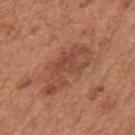* notes — catalogued during a skin exam; not biopsied
* patient — male, in their mid- to late 60s
* body site — the mid back
* image — ~15 mm tile from a whole-body skin photo
* lesion diameter — ~7 mm (longest diameter)
* automated lesion analysis — a lesion area of about 16 mm² and two-axis asymmetry of about 0.4; a color-variation rating of about 3.5/10 and peripheral color asymmetry of about 1